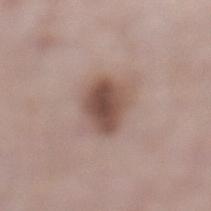Q: Is there a histopathology result?
A: total-body-photography surveillance lesion; no biopsy
Q: Who is the patient?
A: male, aged 38 to 42
Q: What is the anatomic site?
A: the left lower leg
Q: What kind of image is this?
A: ~15 mm crop, total-body skin-cancer survey